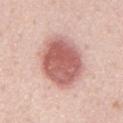| key | value |
|---|---|
| notes | catalogued during a skin exam; not biopsied |
| patient | male, in their mid-50s |
| tile lighting | white-light illumination |
| location | the mid back |
| image source | 15 mm crop, total-body photography |